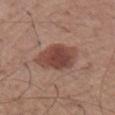Recorded during total-body skin imaging; not selected for excision or biopsy. The lesion's longest dimension is about 5 mm. A lesion tile, about 15 mm wide, cut from a 3D total-body photograph. The lesion is on the right upper arm. The tile uses white-light illumination. A male subject aged around 55.